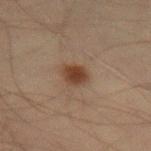Captured during whole-body skin photography for melanoma surveillance; the lesion was not biopsied. A close-up tile cropped from a whole-body skin photograph, about 15 mm across. From the lower back. The patient is a male roughly 70 years of age. The lesion-visualizer software estimated an area of roughly 5 mm² and a shape-asymmetry score of about 0.15 (0 = symmetric). It also reported a border-irregularity rating of about 1.5/10 and peripheral color asymmetry of about 0.5. The analysis additionally found a classifier nevus-likeness of about 100/100. Approximately 2.5 mm at its widest.